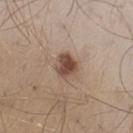Clinical impression:
The lesion was tiled from a total-body skin photograph and was not biopsied.
Clinical summary:
A 15 mm close-up extracted from a 3D total-body photography capture. Captured under white-light illumination. Longest diameter approximately 2.5 mm. A male subject, in their mid-40s. The lesion is on the left thigh. The total-body-photography lesion software estimated a lesion color around L≈47 a*≈18 b*≈25 in CIELAB, roughly 14 lightness units darker than nearby skin, and a normalized border contrast of about 10. The analysis additionally found an automated nevus-likeness rating near 95 out of 100 and a lesion-detection confidence of about 100/100.